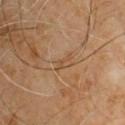  biopsy_status: not biopsied; imaged during a skin examination
  automated_metrics:
    area_mm2_approx: 2.5
    eccentricity: 0.95
    shape_asymmetry: 0.5
    cielab_L: 48
    cielab_a: 17
    cielab_b: 33
    vs_skin_darker_L: 6.0
    nevus_likeness_0_100: 0
    lesion_detection_confidence_0_100: 90
  patient:
    sex: male
    age_approx: 60
  site: upper back
  lesion_size:
    long_diameter_mm_approx: 2.5
  lighting: cross-polarized
  image:
    source: total-body photography crop
    field_of_view_mm: 15